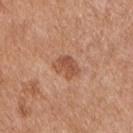{
  "biopsy_status": "not biopsied; imaged during a skin examination",
  "site": "chest",
  "patient": {
    "sex": "male",
    "age_approx": 55
  },
  "lighting": "white-light",
  "image": {
    "source": "total-body photography crop",
    "field_of_view_mm": 15
  },
  "lesion_size": {
    "long_diameter_mm_approx": 3.0
  }
}